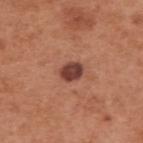Part of a total-body skin-imaging series; this lesion was reviewed on a skin check and was not flagged for biopsy.
Captured under white-light illumination.
This image is a 15 mm lesion crop taken from a total-body photograph.
A male patient about 55 years old.
About 3 mm across.
Located on the upper back.
Automated image analysis of the tile measured an area of roughly 5 mm², a shape eccentricity near 0.6, and two-axis asymmetry of about 0.2. It also reported an automated nevus-likeness rating near 85 out of 100 and lesion-presence confidence of about 100/100.